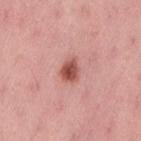biopsy status=no biopsy performed (imaged during a skin exam)
image source=~15 mm tile from a whole-body skin photo
anatomic site=the lower back
subject=female, approximately 50 years of age
tile lighting=white-light